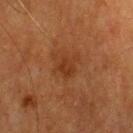| feature | finding |
|---|---|
| biopsy status | total-body-photography surveillance lesion; no biopsy |
| subject | female, in their mid-50s |
| lesion diameter | ≈3 mm |
| anatomic site | the head or neck |
| imaging modality | total-body-photography crop, ~15 mm field of view |